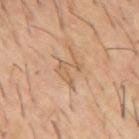Case summary:
* follow-up — no biopsy performed (imaged during a skin exam)
* body site — the mid back
* automated lesion analysis — an eccentricity of roughly 0.6 and a symmetry-axis asymmetry near 0.65; a lesion color around L≈56 a*≈16 b*≈32 in CIELAB, about 7 CIELAB-L* units darker than the surrounding skin, and a lesion-to-skin contrast of about 5 (normalized; higher = more distinct); a nevus-likeness score of about 0/100
* image source — total-body-photography crop, ~15 mm field of view
* patient — male, aged approximately 60
* size — about 4 mm
* lighting — cross-polarized